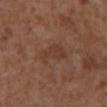The lesion was photographed on a routine skin check and not biopsied; there is no pathology result.
The lesion is on the abdomen.
The lesion's longest dimension is about 4 mm.
Cropped from a total-body skin-imaging series; the visible field is about 15 mm.
The patient is a male aged 73 to 77.